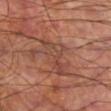biopsy status: catalogued during a skin exam; not biopsied
patient: male, about 60 years old
acquisition: 15 mm crop, total-body photography
site: the right lower leg
lesion size: about 5 mm
image-analysis metrics: an average lesion color of about L≈44 a*≈23 b*≈27 (CIELAB), about 6 CIELAB-L* units darker than the surrounding skin, and a normalized lesion–skin contrast near 5.5; a border-irregularity rating of about 7.5/10, a within-lesion color-variation index near 5/10, and radial color variation of about 1.5; lesion-presence confidence of about 70/100